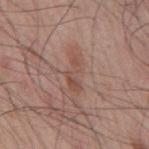| key | value |
|---|---|
| notes | total-body-photography surveillance lesion; no biopsy |
| lighting | white-light |
| anatomic site | the mid back |
| patient | male, aged 53–57 |
| TBP lesion metrics | a lesion color around L≈49 a*≈20 b*≈26 in CIELAB, roughly 7 lightness units darker than nearby skin, and a lesion-to-skin contrast of about 5.5 (normalized; higher = more distinct); a classifier nevus-likeness of about 0/100 |
| lesion diameter | ≈4 mm |
| image source | ~15 mm crop, total-body skin-cancer survey |